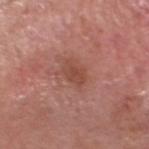<record>
  <biopsy_status>not biopsied; imaged during a skin examination</biopsy_status>
  <automated_metrics>
    <area_mm2_approx>4.0</area_mm2_approx>
    <shape_asymmetry>0.2</shape_asymmetry>
    <vs_skin_darker_L>7.0</vs_skin_darker_L>
    <vs_skin_contrast_norm>6.0</vs_skin_contrast_norm>
    <border_irregularity_0_10>2.5</border_irregularity_0_10>
    <color_variation_0_10>2.0</color_variation_0_10>
    <peripheral_color_asymmetry>0.5</peripheral_color_asymmetry>
    <nevus_likeness_0_100>0</nevus_likeness_0_100>
    <lesion_detection_confidence_0_100>100</lesion_detection_confidence_0_100>
  </automated_metrics>
  <patient>
    <sex>male</sex>
    <age_approx>60</age_approx>
  </patient>
  <site>head or neck</site>
  <lighting>white-light</lighting>
  <image>
    <source>total-body photography crop</source>
    <field_of_view_mm>15</field_of_view_mm>
  </image>
  <lesion_size>
    <long_diameter_mm_approx>2.5</long_diameter_mm_approx>
  </lesion_size>
</record>